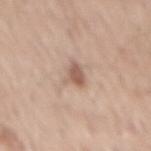Assessment: Part of a total-body skin-imaging series; this lesion was reviewed on a skin check and was not flagged for biopsy.